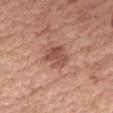The lesion was tiled from a total-body skin photograph and was not biopsied. The patient is a female aged 58 to 62. This is a white-light tile. From the arm. Longest diameter approximately 4 mm. A close-up tile cropped from a whole-body skin photograph, about 15 mm across.